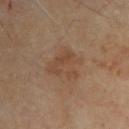Acquisition and patient details: Located on the front of the torso. A 15 mm close-up tile from a total-body photography series done for melanoma screening. The tile uses cross-polarized illumination. The lesion's longest dimension is about 4 mm. A male patient roughly 65 years of age.